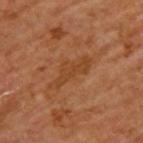{"biopsy_status": "not biopsied; imaged during a skin examination", "image": {"source": "total-body photography crop", "field_of_view_mm": 15}, "site": "back", "patient": {"sex": "male", "age_approx": 65}}